A male subject about 65 years old; imaged with cross-polarized lighting; on the leg; a roughly 15 mm field-of-view crop from a total-body skin photograph.
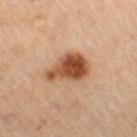Q: What is the histopathologic diagnosis?
A: an intradermal melanocytic nevus (benign)